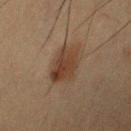Case summary:
* follow-up · catalogued during a skin exam; not biopsied
* size · ≈5 mm
* subject · male, roughly 50 years of age
* illumination · cross-polarized
* site · the right upper arm
* image source · ~15 mm crop, total-body skin-cancer survey